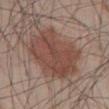<lesion>
<patient>
  <sex>male</sex>
  <age_approx>50</age_approx>
</patient>
<image>
  <source>total-body photography crop</source>
  <field_of_view_mm>15</field_of_view_mm>
</image>
<automated_metrics>
  <cielab_L>46</cielab_L>
  <cielab_a>20</cielab_a>
  <cielab_b>24</cielab_b>
  <vs_skin_darker_L>9.0</vs_skin_darker_L>
  <vs_skin_contrast_norm>7.5</vs_skin_contrast_norm>
  <color_variation_0_10>4.0</color_variation_0_10>
  <peripheral_color_asymmetry>1.5</peripheral_color_asymmetry>
</automated_metrics>
<site>abdomen</site>
<lighting>white-light</lighting>
</lesion>